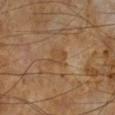Q: Is there a histopathology result?
A: catalogued during a skin exam; not biopsied
Q: What are the patient's age and sex?
A: male, approximately 65 years of age
Q: Automated lesion metrics?
A: an average lesion color of about L≈45 a*≈17 b*≈33 (CIELAB), a lesion–skin lightness drop of about 5, and a normalized lesion–skin contrast near 5; a border-irregularity rating of about 3.5/10, internal color variation of about 1.5 on a 0–10 scale, and a peripheral color-asymmetry measure near 0.5; a nevus-likeness score of about 0/100 and a lesion-detection confidence of about 100/100
Q: What is the lesion's diameter?
A: ~3 mm (longest diameter)
Q: How was this image acquired?
A: total-body-photography crop, ~15 mm field of view
Q: What lighting was used for the tile?
A: cross-polarized illumination
Q: Lesion location?
A: the left lower leg